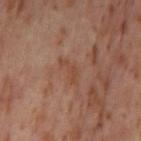Q: Who is the patient?
A: female, in their mid-50s
Q: What is the anatomic site?
A: the left thigh
Q: How was this image acquired?
A: total-body-photography crop, ~15 mm field of view